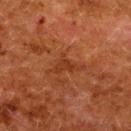  biopsy_status: not biopsied; imaged during a skin examination
  site: upper back
  patient:
    sex: female
    age_approx: 50
  lesion_size:
    long_diameter_mm_approx: 4.0
  image:
    source: total-body photography crop
    field_of_view_mm: 15